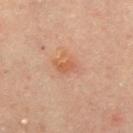Assessment:
The lesion was tiled from a total-body skin photograph and was not biopsied.
Background:
This image is a 15 mm lesion crop taken from a total-body photograph. From the abdomen. A male subject, aged 63–67. Imaged with cross-polarized lighting. About 3 mm across.